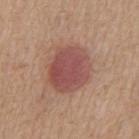The lesion was tiled from a total-body skin photograph and was not biopsied. Captured under white-light illumination. The total-body-photography lesion software estimated a footprint of about 19 mm² and two-axis asymmetry of about 0.1. And it measured a border-irregularity index near 1/10, a within-lesion color-variation index near 3.5/10, and a peripheral color-asymmetry measure near 1. It also reported an automated nevus-likeness rating near 95 out of 100. The lesion is located on the front of the torso. The subject is a male in their 70s. Longest diameter approximately 5 mm. This image is a 15 mm lesion crop taken from a total-body photograph.